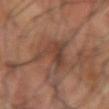Assessment: Recorded during total-body skin imaging; not selected for excision or biopsy. Background: A 15 mm close-up extracted from a 3D total-body photography capture. The lesion-visualizer software estimated a lesion–skin lightness drop of about 10. On the left forearm. The recorded lesion diameter is about 8 mm. Imaged with cross-polarized lighting. The subject is a male aged 63–67.